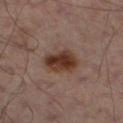Clinical impression:
Imaged during a routine full-body skin examination; the lesion was not biopsied and no histopathology is available.